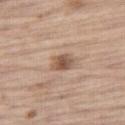workup = catalogued during a skin exam; not biopsied | patient = male, roughly 70 years of age | imaging modality = ~15 mm crop, total-body skin-cancer survey | TBP lesion metrics = an area of roughly 5 mm², an eccentricity of roughly 0.7, and a symmetry-axis asymmetry near 0.25; an average lesion color of about L≈54 a*≈18 b*≈28 (CIELAB), about 12 CIELAB-L* units darker than the surrounding skin, and a normalized border contrast of about 8.5; a border-irregularity index near 2/10, a color-variation rating of about 5/10, and peripheral color asymmetry of about 1.5; an automated nevus-likeness rating near 90 out of 100 and a lesion-detection confidence of about 100/100 | illumination = white-light illumination | location = the right thigh | diameter = about 2.5 mm.